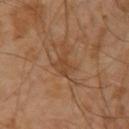Q: Is there a histopathology result?
A: total-body-photography surveillance lesion; no biopsy
Q: What are the patient's age and sex?
A: male, aged 28 to 32
Q: What kind of image is this?
A: total-body-photography crop, ~15 mm field of view
Q: Lesion size?
A: ≈3.5 mm
Q: How was the tile lit?
A: cross-polarized illumination
Q: Where on the body is the lesion?
A: the left upper arm